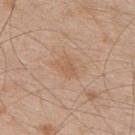| feature | finding |
|---|---|
| biopsy status | total-body-photography surveillance lesion; no biopsy |
| image source | ~15 mm tile from a whole-body skin photo |
| subject | male, aged approximately 50 |
| anatomic site | the upper back |